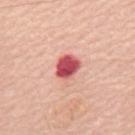{
  "biopsy_status": "not biopsied; imaged during a skin examination",
  "lighting": "white-light",
  "site": "upper back",
  "image": {
    "source": "total-body photography crop",
    "field_of_view_mm": 15
  },
  "patient": {
    "sex": "male",
    "age_approx": 70
  },
  "lesion_size": {
    "long_diameter_mm_approx": 3.0
  }
}